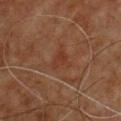Clinical impression:
This lesion was catalogued during total-body skin photography and was not selected for biopsy.
Context:
From the chest. A lesion tile, about 15 mm wide, cut from a 3D total-body photograph. About 3 mm across. A male subject about 60 years old. This is a cross-polarized tile.